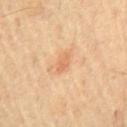Case summary:
– notes · imaged on a skin check; not biopsied
– patient · male, aged 63 to 67
– site · the chest
– image · ~15 mm tile from a whole-body skin photo